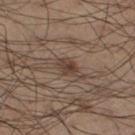<lesion>
<biopsy_status>not biopsied; imaged during a skin examination</biopsy_status>
<lesion_size>
  <long_diameter_mm_approx>2.5</long_diameter_mm_approx>
</lesion_size>
<image>
  <source>total-body photography crop</source>
  <field_of_view_mm>15</field_of_view_mm>
</image>
<lighting>white-light</lighting>
<automated_metrics>
  <area_mm2_approx>4.5</area_mm2_approx>
  <eccentricity>0.65</eccentricity>
  <cielab_L>41</cielab_L>
  <cielab_a>15</cielab_a>
  <cielab_b>24</cielab_b>
  <vs_skin_darker_L>9.0</vs_skin_darker_L>
  <vs_skin_contrast_norm>8.0</vs_skin_contrast_norm>
</automated_metrics>
<patient>
  <sex>male</sex>
  <age_approx>55</age_approx>
</patient>
<site>left lower leg</site>
</lesion>